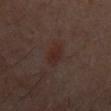Assessment:
Imaged during a routine full-body skin examination; the lesion was not biopsied and no histopathology is available.
Context:
Captured under cross-polarized illumination. The lesion-visualizer software estimated an average lesion color of about L≈23 a*≈16 b*≈19 (CIELAB), a lesion–skin lightness drop of about 5, and a lesion-to-skin contrast of about 7 (normalized; higher = more distinct). The analysis additionally found border irregularity of about 2.5 on a 0–10 scale, internal color variation of about 1.5 on a 0–10 scale, and a peripheral color-asymmetry measure near 0.5. The recorded lesion diameter is about 3 mm. A male subject, roughly 60 years of age. A 15 mm crop from a total-body photograph taken for skin-cancer surveillance. From the mid back.